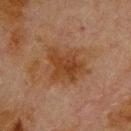Clinical summary: A 15 mm crop from a total-body photograph taken for skin-cancer surveillance. On the back. A male subject, approximately 80 years of age.